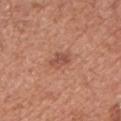workup: catalogued during a skin exam; not biopsied | diameter: ~2.5 mm (longest diameter) | image-analysis metrics: a lesion area of about 4 mm², a shape eccentricity near 0.75, and a shape-asymmetry score of about 0.25 (0 = symmetric); a lesion color around L≈51 a*≈25 b*≈30 in CIELAB, roughly 9 lightness units darker than nearby skin, and a normalized border contrast of about 6; border irregularity of about 2.5 on a 0–10 scale, a within-lesion color-variation index near 2.5/10, and radial color variation of about 1 | site: the left upper arm | subject: male, in their mid-70s | acquisition: 15 mm crop, total-body photography | lighting: white-light.